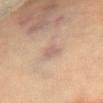workup = catalogued during a skin exam; not biopsied
body site = the chest
lesion size = ≈2.5 mm
imaging modality = ~15 mm tile from a whole-body skin photo
patient = female, aged approximately 80